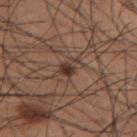notes: no biopsy performed (imaged during a skin exam); illumination: white-light; TBP lesion metrics: a border-irregularity rating of about 5/10 and a color-variation rating of about 3/10; subject: male, aged 33 to 37; image source: total-body-photography crop, ~15 mm field of view; lesion diameter: ≈3 mm; location: the right upper arm.